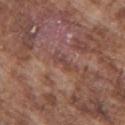Part of a total-body skin-imaging series; this lesion was reviewed on a skin check and was not flagged for biopsy.
Imaged with white-light lighting.
A male patient roughly 75 years of age.
A lesion tile, about 15 mm wide, cut from a 3D total-body photograph.
Located on the right upper arm.
Approximately 2.5 mm at its widest.